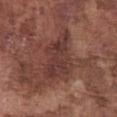Notes:
– follow-up · imaged on a skin check; not biopsied
– lighting · white-light
– subject · male, roughly 75 years of age
– image-analysis metrics · a within-lesion color-variation index near 4/10 and a peripheral color-asymmetry measure near 1; a nevus-likeness score of about 0/100 and a lesion-detection confidence of about 70/100
– diameter · ~6.5 mm (longest diameter)
– location · the chest
– acquisition · ~15 mm tile from a whole-body skin photo